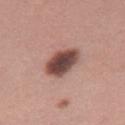This lesion was catalogued during total-body skin photography and was not selected for biopsy. About 5 mm across. Imaged with white-light lighting. The total-body-photography lesion software estimated a footprint of about 11 mm², an eccentricity of roughly 0.85, and a symmetry-axis asymmetry near 0.2. The software also gave a nevus-likeness score of about 30/100. A female patient in their 30s. A 15 mm crop from a total-body photograph taken for skin-cancer surveillance. Located on the right thigh.